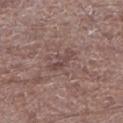Q: Was a biopsy performed?
A: no biopsy performed (imaged during a skin exam)